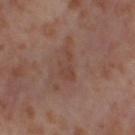Captured during whole-body skin photography for melanoma surveillance; the lesion was not biopsied. On the left thigh. Cropped from a whole-body photographic skin survey; the tile spans about 15 mm. The total-body-photography lesion software estimated an eccentricity of roughly 0.85. The analysis additionally found a mean CIELAB color near L≈43 a*≈19 b*≈26, about 6 CIELAB-L* units darker than the surrounding skin, and a normalized border contrast of about 5.5. The analysis additionally found a classifier nevus-likeness of about 0/100 and lesion-presence confidence of about 100/100. The lesion's longest dimension is about 3.5 mm. A female patient, aged approximately 55.